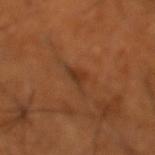Automated image analysis of the tile measured a lesion area of about 3 mm², an eccentricity of roughly 0.8, and a symmetry-axis asymmetry near 0.5. The analysis additionally found an average lesion color of about L≈35 a*≈23 b*≈33 (CIELAB), a lesion–skin lightness drop of about 7, and a lesion-to-skin contrast of about 6 (normalized; higher = more distinct). The software also gave a classifier nevus-likeness of about 0/100. The patient is a male in their mid- to late 60s. On the left lower leg. The lesion's longest dimension is about 2.5 mm. Cropped from a total-body skin-imaging series; the visible field is about 15 mm. This is a cross-polarized tile.